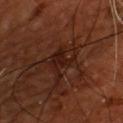Findings:
- biopsy status: catalogued during a skin exam; not biopsied
- lesion diameter: ≈5 mm
- body site: the chest
- subject: male, roughly 55 years of age
- tile lighting: cross-polarized illumination
- image: total-body-photography crop, ~15 mm field of view